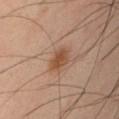Clinical impression: This lesion was catalogued during total-body skin photography and was not selected for biopsy. Clinical summary: Longest diameter approximately 2.5 mm. A 15 mm close-up extracted from a 3D total-body photography capture. A male subject approximately 40 years of age. From the left upper arm.